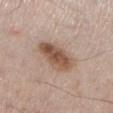Assessment: No biopsy was performed on this lesion — it was imaged during a full skin examination and was not determined to be concerning. Background: This is a white-light tile. Longest diameter approximately 5 mm. A close-up tile cropped from a whole-body skin photograph, about 15 mm across. On the left lower leg. A male subject, aged around 60.